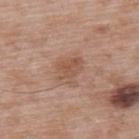Captured during whole-body skin photography for melanoma surveillance; the lesion was not biopsied.
Approximately 3.5 mm at its widest.
Cropped from a total-body skin-imaging series; the visible field is about 15 mm.
The total-body-photography lesion software estimated a footprint of about 6 mm², an outline eccentricity of about 0.65 (0 = round, 1 = elongated), and two-axis asymmetry of about 0.35.
The tile uses white-light illumination.
On the upper back.
A male patient aged 48 to 52.